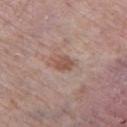Assessment:
The lesion was photographed on a routine skin check and not biopsied; there is no pathology result.
Context:
A male patient, approximately 85 years of age or older. Captured under white-light illumination. A roughly 15 mm field-of-view crop from a total-body skin photograph. Approximately 2.5 mm at its widest. Located on the left thigh. An algorithmic analysis of the crop reported a mean CIELAB color near L≈52 a*≈19 b*≈26 and a normalized lesion–skin contrast near 7. It also reported peripheral color asymmetry of about 1. The software also gave a nevus-likeness score of about 10/100.